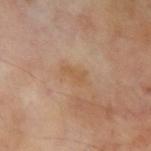  biopsy_status: not biopsied; imaged during a skin examination
  automated_metrics:
    vs_skin_contrast_norm: 5.5
    nevus_likeness_0_100: 0
  site: left upper arm
  image:
    source: total-body photography crop
    field_of_view_mm: 15
  lighting: cross-polarized
  lesion_size:
    long_diameter_mm_approx: 3.0
  patient:
    sex: male
    age_approx: 70